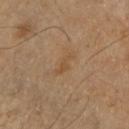{
  "biopsy_status": "not biopsied; imaged during a skin examination",
  "site": "right forearm",
  "image": {
    "source": "total-body photography crop",
    "field_of_view_mm": 15
  },
  "lesion_size": {
    "long_diameter_mm_approx": 3.0
  },
  "lighting": "cross-polarized",
  "patient": {
    "sex": "female",
    "age_approx": 60
  }
}